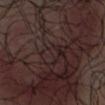Clinical impression:
Captured during whole-body skin photography for melanoma surveillance; the lesion was not biopsied.
Image and clinical context:
From the chest. Measured at roughly 23 mm in maximum diameter. A 15 mm close-up tile from a total-body photography series done for melanoma screening. The subject is a male aged around 30. An algorithmic analysis of the crop reported an average lesion color of about L≈25 a*≈15 b*≈15 (CIELAB), roughly 8 lightness units darker than nearby skin, and a lesion-to-skin contrast of about 9 (normalized; higher = more distinct). And it measured an automated nevus-likeness rating near 0 out of 100 and a detector confidence of about 70 out of 100 that the crop contains a lesion.